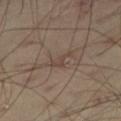follow-up: no biopsy performed (imaged during a skin exam)
site: the leg
subject: male, aged 38 to 42
image source: ~15 mm crop, total-body skin-cancer survey